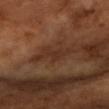Part of a total-body skin-imaging series; this lesion was reviewed on a skin check and was not flagged for biopsy. The subject is a female in their 70s. A 15 mm close-up tile from a total-body photography series done for melanoma screening. On the right forearm.